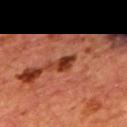follow-up — catalogued during a skin exam; not biopsied | image — ~15 mm crop, total-body skin-cancer survey | illumination — cross-polarized illumination | subject — male, aged approximately 65 | site — the mid back.